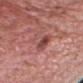Impression: Recorded during total-body skin imaging; not selected for excision or biopsy. Clinical summary: A close-up tile cropped from a whole-body skin photograph, about 15 mm across. The subject is a male aged around 60. Automated tile analysis of the lesion measured a lesion area of about 7 mm² and an outline eccentricity of about 0.85 (0 = round, 1 = elongated). It also reported roughly 9 lightness units darker than nearby skin. And it measured border irregularity of about 5 on a 0–10 scale, a color-variation rating of about 6/10, and peripheral color asymmetry of about 2. The lesion is located on the head or neck. The tile uses white-light illumination.